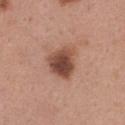This lesion was catalogued during total-body skin photography and was not selected for biopsy.